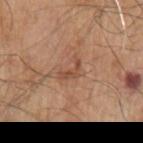Captured during whole-body skin photography for melanoma surveillance; the lesion was not biopsied. The lesion's longest dimension is about 3 mm. Captured under white-light illumination. Automated image analysis of the tile measured a lesion area of about 3 mm² and two-axis asymmetry of about 0.65. It also reported a border-irregularity index near 7/10, a color-variation rating of about 0/10, and a peripheral color-asymmetry measure near 0. The lesion is on the left upper arm. A roughly 15 mm field-of-view crop from a total-body skin photograph. A male subject aged around 80.